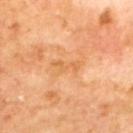Imaged during a routine full-body skin examination; the lesion was not biopsied and no histopathology is available.
Measured at roughly 3.5 mm in maximum diameter.
Captured under cross-polarized illumination.
On the upper back.
A close-up tile cropped from a whole-body skin photograph, about 15 mm across.
A male subject, roughly 70 years of age.
The total-body-photography lesion software estimated an average lesion color of about L≈64 a*≈25 b*≈44 (CIELAB), roughly 6 lightness units darker than nearby skin, and a normalized lesion–skin contrast near 5.